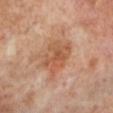workup: no biopsy performed (imaged during a skin exam) | image: total-body-photography crop, ~15 mm field of view | TBP lesion metrics: a lesion area of about 13 mm², a shape eccentricity near 0.65, and two-axis asymmetry of about 0.2; border irregularity of about 2.5 on a 0–10 scale, a color-variation rating of about 5/10, and peripheral color asymmetry of about 2; lesion-presence confidence of about 100/100 | body site: the left lower leg | lighting: cross-polarized | lesion size: ~5 mm (longest diameter) | subject: male, about 70 years old.